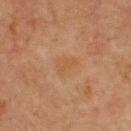No biopsy was performed on this lesion — it was imaged during a full skin examination and was not determined to be concerning.
A roughly 15 mm field-of-view crop from a total-body skin photograph.
A male patient approximately 60 years of age.
An algorithmic analysis of the crop reported two-axis asymmetry of about 0.4. The software also gave an average lesion color of about L≈44 a*≈17 b*≈32 (CIELAB), a lesion–skin lightness drop of about 4, and a normalized lesion–skin contrast near 4.5. The software also gave a border-irregularity rating of about 3.5/10 and internal color variation of about 1 on a 0–10 scale.
From the back.
Approximately 3 mm at its widest.